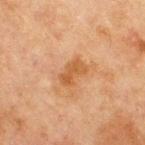Impression:
Captured during whole-body skin photography for melanoma surveillance; the lesion was not biopsied.
Acquisition and patient details:
On the chest. Longest diameter approximately 3 mm. The subject is a male aged approximately 65. A roughly 15 mm field-of-view crop from a total-body skin photograph.